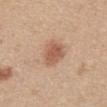<lesion>
<biopsy_status>not biopsied; imaged during a skin examination</biopsy_status>
<site>abdomen</site>
<lesion_size>
  <long_diameter_mm_approx>3.5</long_diameter_mm_approx>
</lesion_size>
<lighting>white-light</lighting>
<image>
  <source>total-body photography crop</source>
  <field_of_view_mm>15</field_of_view_mm>
</image>
<patient>
  <sex>female</sex>
  <age_approx>45</age_approx>
</patient>
</lesion>